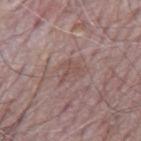Notes:
- notes · catalogued during a skin exam; not biopsied
- illumination · white-light illumination
- automated metrics · an area of roughly 3 mm², an outline eccentricity of about 0.8 (0 = round, 1 = elongated), and two-axis asymmetry of about 0.75; a mean CIELAB color near L≈50 a*≈18 b*≈21, a lesion–skin lightness drop of about 6, and a normalized border contrast of about 5; a border-irregularity index near 7/10
- patient · male, approximately 65 years of age
- site · the mid back
- acquisition · ~15 mm tile from a whole-body skin photo